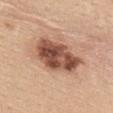Captured under white-light illumination.
From the upper back.
Longest diameter approximately 6.5 mm.
A close-up tile cropped from a whole-body skin photograph, about 15 mm across.
A female patient, roughly 45 years of age.